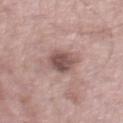Q: Is there a histopathology result?
A: imaged on a skin check; not biopsied
Q: What are the patient's age and sex?
A: male, approximately 55 years of age
Q: What is the anatomic site?
A: the right lower leg
Q: Automated lesion metrics?
A: a shape eccentricity near 0.6 and two-axis asymmetry of about 0.15
Q: Illumination type?
A: white-light
Q: What kind of image is this?
A: ~15 mm tile from a whole-body skin photo